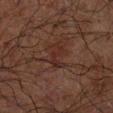No biopsy was performed on this lesion — it was imaged during a full skin examination and was not determined to be concerning.
The lesion's longest dimension is about 3 mm.
The tile uses cross-polarized illumination.
From the left forearm.
The subject is a male aged 68 to 72.
A region of skin cropped from a whole-body photographic capture, roughly 15 mm wide.